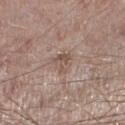The lesion was tiled from a total-body skin photograph and was not biopsied.
Located on the left lower leg.
A male subject roughly 65 years of age.
Cropped from a whole-body photographic skin survey; the tile spans about 15 mm.